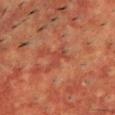Q: Was a biopsy performed?
A: imaged on a skin check; not biopsied
Q: What is the imaging modality?
A: ~15 mm crop, total-body skin-cancer survey
Q: How large is the lesion?
A: about 3.5 mm
Q: What are the patient's age and sex?
A: male, aged 53–57
Q: What did automated image analysis measure?
A: an area of roughly 4 mm² and an eccentricity of roughly 0.9; a lesion color around L≈40 a*≈28 b*≈29 in CIELAB, roughly 5 lightness units darker than nearby skin, and a normalized lesion–skin contrast near 4.5
Q: Illumination type?
A: cross-polarized
Q: What is the anatomic site?
A: the upper back